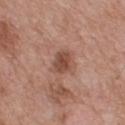Notes:
* workup — catalogued during a skin exam; not biopsied
* size — about 3 mm
* image — total-body-photography crop, ~15 mm field of view
* subject — male, aged 63 to 67
* image-analysis metrics — a symmetry-axis asymmetry near 0.2; a detector confidence of about 100 out of 100 that the crop contains a lesion
* body site — the upper back